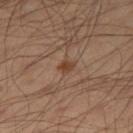The lesion was tiled from a total-body skin photograph and was not biopsied. The patient is a male approximately 55 years of age. Located on the right thigh. A 15 mm close-up extracted from a 3D total-body photography capture.